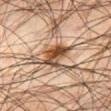Assessment:
This lesion was catalogued during total-body skin photography and was not selected for biopsy.
Background:
A male subject aged 43 to 47. On the leg. A 15 mm close-up extracted from a 3D total-body photography capture.